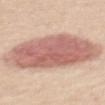notes = imaged on a skin check; not biopsied
anatomic site = the mid back
size = ~8.5 mm (longest diameter)
subject = female, aged 48–52
automated lesion analysis = a mean CIELAB color near L≈62 a*≈23 b*≈26, about 14 CIELAB-L* units darker than the surrounding skin, and a normalized lesion–skin contrast near 8.5; radial color variation of about 1.5
acquisition = total-body-photography crop, ~15 mm field of view